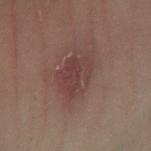Q: What kind of image is this?
A: 15 mm crop, total-body photography
Q: Lesion location?
A: the left leg
Q: How was the tile lit?
A: cross-polarized
Q: Who is the patient?
A: female, approximately 30 years of age
Q: Lesion size?
A: ≈5 mm
Q: What did automated image analysis measure?
A: a border-irregularity index near 2/10 and a peripheral color-asymmetry measure near 1